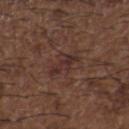<case>
<biopsy_status>not biopsied; imaged during a skin examination</biopsy_status>
</case>